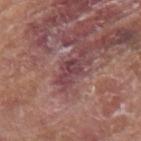location = the arm
acquisition = ~15 mm tile from a whole-body skin photo
lighting = white-light
patient = male, roughly 80 years of age
lesion diameter = about 3 mm
image-analysis metrics = a border-irregularity rating of about 5.5/10, internal color variation of about 3.5 on a 0–10 scale, and peripheral color asymmetry of about 1